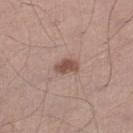workup: total-body-photography surveillance lesion; no biopsy | TBP lesion metrics: a footprint of about 4 mm² and an outline eccentricity of about 0.75 (0 = round, 1 = elongated); a mean CIELAB color near L≈51 a*≈19 b*≈25, about 12 CIELAB-L* units darker than the surrounding skin, and a lesion-to-skin contrast of about 8.5 (normalized; higher = more distinct); a lesion-detection confidence of about 100/100 | lighting: white-light illumination | location: the leg | patient: male, roughly 45 years of age | size: ≈3 mm | imaging modality: ~15 mm crop, total-body skin-cancer survey.